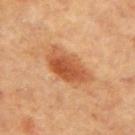{"biopsy_status": "not biopsied; imaged during a skin examination", "site": "arm", "image": {"source": "total-body photography crop", "field_of_view_mm": 15}, "patient": {"sex": "female", "age_approx": 65}, "lighting": "cross-polarized", "lesion_size": {"long_diameter_mm_approx": 5.5}}